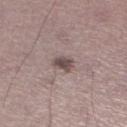follow-up: total-body-photography surveillance lesion; no biopsy | imaging modality: ~15 mm tile from a whole-body skin photo | illumination: white-light illumination | anatomic site: the right lower leg | subject: male, approximately 45 years of age | image-analysis metrics: a lesion area of about 4.5 mm², an eccentricity of roughly 0.75, and a shape-asymmetry score of about 0.25 (0 = symmetric); roughly 12 lightness units darker than nearby skin and a normalized lesion–skin contrast near 8.5; an automated nevus-likeness rating near 70 out of 100 | lesion diameter: ≈3 mm.